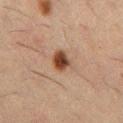  patient:
    sex: male
    age_approx: 50
  lesion_size:
    long_diameter_mm_approx: 2.5
  site: chest
  image:
    source: total-body photography crop
    field_of_view_mm: 15